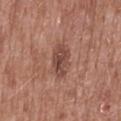Assessment:
Imaged during a routine full-body skin examination; the lesion was not biopsied and no histopathology is available.
Background:
Captured under white-light illumination. From the mid back. A close-up tile cropped from a whole-body skin photograph, about 15 mm across. A male subject, aged 48–52. The lesion's longest dimension is about 4 mm.